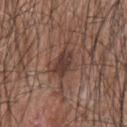| key | value |
|---|---|
| biopsy status | imaged on a skin check; not biopsied |
| illumination | white-light illumination |
| body site | the upper back |
| image source | ~15 mm crop, total-body skin-cancer survey |
| size | ≈3 mm |
| patient | male, roughly 45 years of age |